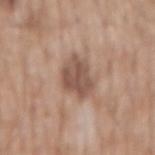notes: total-body-photography surveillance lesion; no biopsy | automated lesion analysis: an average lesion color of about L≈52 a*≈18 b*≈26 (CIELAB), a lesion–skin lightness drop of about 11, and a normalized lesion–skin contrast near 8; a border-irregularity index near 2.5/10, internal color variation of about 3.5 on a 0–10 scale, and radial color variation of about 1; a classifier nevus-likeness of about 30/100 and lesion-presence confidence of about 100/100 | acquisition: total-body-photography crop, ~15 mm field of view | subject: male, aged 58 to 62 | anatomic site: the abdomen.